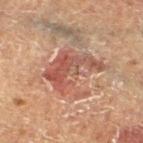Findings:
– notes · imaged on a skin check; not biopsied
– body site · the leg
– TBP lesion metrics · an average lesion color of about L≈48 a*≈22 b*≈26 (CIELAB), roughly 9 lightness units darker than nearby skin, and a lesion-to-skin contrast of about 7 (normalized; higher = more distinct); border irregularity of about 7.5 on a 0–10 scale, a color-variation rating of about 6.5/10, and peripheral color asymmetry of about 2.5
– size · ~6.5 mm (longest diameter)
– patient · male, aged around 65
– lighting · cross-polarized illumination
– acquisition · ~15 mm crop, total-body skin-cancer survey